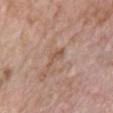Assessment:
The lesion was photographed on a routine skin check and not biopsied; there is no pathology result.
Clinical summary:
A 15 mm close-up extracted from a 3D total-body photography capture. The lesion is located on the front of the torso. A female patient approximately 75 years of age.